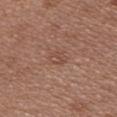Notes:
* biopsy status · imaged on a skin check; not biopsied
* location · the chest
* acquisition · ~15 mm crop, total-body skin-cancer survey
* size · ~2.5 mm (longest diameter)
* image-analysis metrics · a shape eccentricity near 0.75 and a shape-asymmetry score of about 0.3 (0 = symmetric); a lesion color around L≈48 a*≈21 b*≈27 in CIELAB, a lesion–skin lightness drop of about 6, and a normalized border contrast of about 4.5; a border-irregularity index near 2.5/10, a within-lesion color-variation index near 3/10, and peripheral color asymmetry of about 1.5
* subject · female, roughly 40 years of age
* tile lighting · white-light